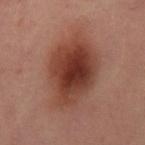Q: Is there a histopathology result?
A: total-body-photography surveillance lesion; no biopsy
Q: Lesion location?
A: the right thigh
Q: What is the imaging modality?
A: 15 mm crop, total-body photography
Q: Patient demographics?
A: female, aged 38–42